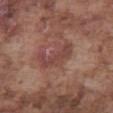Q: Lesion location?
A: the abdomen
Q: How was this image acquired?
A: ~15 mm tile from a whole-body skin photo
Q: What are the patient's age and sex?
A: male, in their mid- to late 70s